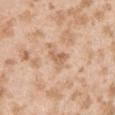{
  "biopsy_status": "not biopsied; imaged during a skin examination",
  "lighting": "white-light",
  "lesion_size": {
    "long_diameter_mm_approx": 3.5
  },
  "patient": {
    "sex": "female",
    "age_approx": 25
  },
  "site": "left upper arm",
  "automated_metrics": {
    "area_mm2_approx": 4.5,
    "eccentricity": 0.85,
    "shape_asymmetry": 0.55,
    "peripheral_color_asymmetry": 1.0,
    "nevus_likeness_0_100": 0,
    "lesion_detection_confidence_0_100": 100
  },
  "image": {
    "source": "total-body photography crop",
    "field_of_view_mm": 15
  }
}